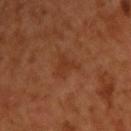Q: Was this lesion biopsied?
A: total-body-photography surveillance lesion; no biopsy
Q: How was this image acquired?
A: ~15 mm tile from a whole-body skin photo
Q: What are the patient's age and sex?
A: male, roughly 50 years of age
Q: Lesion location?
A: the upper back
Q: What lighting was used for the tile?
A: cross-polarized illumination
Q: What is the lesion's diameter?
A: ~3.5 mm (longest diameter)
Q: Automated lesion metrics?
A: a lesion area of about 5.5 mm², a shape eccentricity near 0.5, and a symmetry-axis asymmetry near 0.5; an average lesion color of about L≈35 a*≈24 b*≈33 (CIELAB), a lesion–skin lightness drop of about 5, and a normalized lesion–skin contrast near 5; internal color variation of about 1.5 on a 0–10 scale and radial color variation of about 0.5